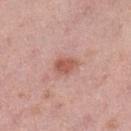subject: female, aged 48 to 52
body site: the left lower leg
image: ~15 mm crop, total-body skin-cancer survey
lesion diameter: ≈2.5 mm